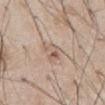Clinical impression: This lesion was catalogued during total-body skin photography and was not selected for biopsy. Context: Located on the abdomen. A 15 mm close-up extracted from a 3D total-body photography capture. A male patient, aged around 60.